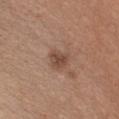Notes:
* follow-up — total-body-photography surveillance lesion; no biopsy
* image — total-body-photography crop, ~15 mm field of view
* subject — female, aged 38 to 42
* site — the chest
* size — about 2.5 mm
* tile lighting — white-light